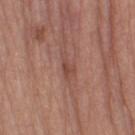Impression: Part of a total-body skin-imaging series; this lesion was reviewed on a skin check and was not flagged for biopsy. Acquisition and patient details: The lesion is on the right thigh. Imaged with white-light lighting. A region of skin cropped from a whole-body photographic capture, roughly 15 mm wide. About 3 mm across. A female subject, roughly 65 years of age.